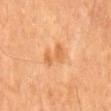biopsy_status: not biopsied; imaged during a skin examination
image:
  source: total-body photography crop
  field_of_view_mm: 15
patient:
  sex: male
  age_approx: 65
site: mid back
lesion_size:
  long_diameter_mm_approx: 4.0